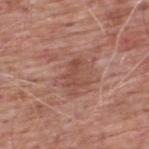<lesion>
  <biopsy_status>not biopsied; imaged during a skin examination</biopsy_status>
  <image>
    <source>total-body photography crop</source>
    <field_of_view_mm>15</field_of_view_mm>
  </image>
  <lighting>white-light</lighting>
  <patient>
    <sex>male</sex>
    <age_approx>60</age_approx>
  </patient>
  <site>upper back</site>
  <automated_metrics>
    <cielab_L>48</cielab_L>
    <cielab_a>23</cielab_a>
    <cielab_b>27</cielab_b>
    <border_irregularity_0_10>7.5</border_irregularity_0_10>
    <color_variation_0_10>0.5</color_variation_0_10>
    <peripheral_color_asymmetry>0.0</peripheral_color_asymmetry>
  </automated_metrics>
</lesion>